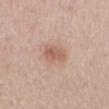This lesion was catalogued during total-body skin photography and was not selected for biopsy. A 15 mm close-up tile from a total-body photography series done for melanoma screening. From the mid back. The recorded lesion diameter is about 3 mm. The patient is a female aged 43–47. The tile uses white-light illumination.